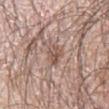Q: What is the lesion's diameter?
A: ~3.5 mm (longest diameter)
Q: Patient demographics?
A: male, aged 63 to 67
Q: What did automated image analysis measure?
A: an area of roughly 4.5 mm², an outline eccentricity of about 0.85 (0 = round, 1 = elongated), and a shape-asymmetry score of about 0.35 (0 = symmetric); a border-irregularity rating of about 3.5/10, a color-variation rating of about 2.5/10, and peripheral color asymmetry of about 1; a classifier nevus-likeness of about 0/100 and a detector confidence of about 60 out of 100 that the crop contains a lesion
Q: Where on the body is the lesion?
A: the arm
Q: What kind of image is this?
A: ~15 mm tile from a whole-body skin photo
Q: How was the tile lit?
A: white-light illumination